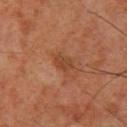Clinical impression: This lesion was catalogued during total-body skin photography and was not selected for biopsy. Clinical summary: From the upper back. A region of skin cropped from a whole-body photographic capture, roughly 15 mm wide. This is a cross-polarized tile. The lesion-visualizer software estimated border irregularity of about 1.5 on a 0–10 scale and internal color variation of about 2 on a 0–10 scale. A male patient aged 58 to 62. Approximately 2.5 mm at its widest.